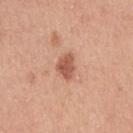Q: Was a biopsy performed?
A: catalogued during a skin exam; not biopsied
Q: Where on the body is the lesion?
A: the right upper arm
Q: Automated lesion metrics?
A: a lesion area of about 5.5 mm², a shape eccentricity near 0.75, and two-axis asymmetry of about 0.3; an average lesion color of about L≈56 a*≈25 b*≈31 (CIELAB) and roughly 13 lightness units darker than nearby skin; a within-lesion color-variation index near 2.5/10 and radial color variation of about 1; an automated nevus-likeness rating near 45 out of 100 and a detector confidence of about 100 out of 100 that the crop contains a lesion
Q: Lesion size?
A: ~3 mm (longest diameter)
Q: What are the patient's age and sex?
A: female, aged 43 to 47
Q: How was this image acquired?
A: total-body-photography crop, ~15 mm field of view